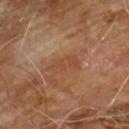Clinical impression:
Part of a total-body skin-imaging series; this lesion was reviewed on a skin check and was not flagged for biopsy.
Acquisition and patient details:
A 15 mm close-up extracted from a 3D total-body photography capture. The lesion is on the chest. The patient is a male aged 58–62. Imaged with cross-polarized lighting. An algorithmic analysis of the crop reported an area of roughly 5.5 mm². The software also gave a mean CIELAB color near L≈44 a*≈21 b*≈32, a lesion–skin lightness drop of about 6, and a normalized border contrast of about 5.5. It also reported a border-irregularity index near 4/10 and a within-lesion color-variation index near 2/10.